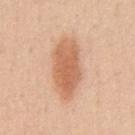{
  "biopsy_status": "not biopsied; imaged during a skin examination",
  "image": {
    "source": "total-body photography crop",
    "field_of_view_mm": 15
  },
  "site": "chest",
  "lighting": "white-light",
  "patient": {
    "sex": "male",
    "age_approx": 50
  }
}